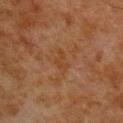biopsy status: catalogued during a skin exam; not biopsied
image-analysis metrics: an area of roughly 3 mm² and an outline eccentricity of about 0.9 (0 = round, 1 = elongated); a nevus-likeness score of about 0/100 and lesion-presence confidence of about 100/100
subject: male, approximately 80 years of age
illumination: cross-polarized illumination
lesion diameter: ~3 mm (longest diameter)
site: the upper back
acquisition: 15 mm crop, total-body photography